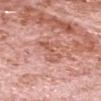<tbp_lesion>
  <biopsy_status>not biopsied; imaged during a skin examination</biopsy_status>
  <image>
    <source>total-body photography crop</source>
    <field_of_view_mm>15</field_of_view_mm>
  </image>
  <patient>
    <sex>male</sex>
    <age_approx>80</age_approx>
  </patient>
  <lesion_size>
    <long_diameter_mm_approx>5.0</long_diameter_mm_approx>
  </lesion_size>
  <site>head or neck</site>
  <lighting>white-light</lighting>
  <automated_metrics>
    <cielab_L>58</cielab_L>
    <cielab_a>26</cielab_a>
    <cielab_b>29</cielab_b>
    <vs_skin_darker_L>9.0</vs_skin_darker_L>
    <vs_skin_contrast_norm>6.0</vs_skin_contrast_norm>
    <border_irregularity_0_10>8.0</border_irregularity_0_10>
    <color_variation_0_10>2.5</color_variation_0_10>
    <peripheral_color_asymmetry>1.0</peripheral_color_asymmetry>
  </automated_metrics>
</tbp_lesion>